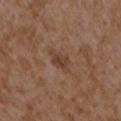The lesion was photographed on a routine skin check and not biopsied; there is no pathology result.
The lesion is on the left forearm.
A female patient aged around 35.
A close-up tile cropped from a whole-body skin photograph, about 15 mm across.
Imaged with white-light lighting.